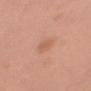<tbp_lesion>
  <biopsy_status>not biopsied; imaged during a skin examination</biopsy_status>
  <lighting>white-light</lighting>
  <image>
    <source>total-body photography crop</source>
    <field_of_view_mm>15</field_of_view_mm>
  </image>
  <lesion_size>
    <long_diameter_mm_approx>3.0</long_diameter_mm_approx>
  </lesion_size>
  <site>arm</site>
  <patient>
    <sex>female</sex>
    <age_approx>65</age_approx>
  </patient>
  <automated_metrics>
    <border_irregularity_0_10>2.5</border_irregularity_0_10>
    <color_variation_0_10>2.0</color_variation_0_10>
    <peripheral_color_asymmetry>1.0</peripheral_color_asymmetry>
  </automated_metrics>
</tbp_lesion>